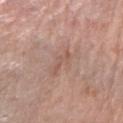lighting = white-light illumination
image = 15 mm crop, total-body photography
patient = female, aged 58–62
size = ~3 mm (longest diameter)
automated lesion analysis = a lesion area of about 2.5 mm², an eccentricity of roughly 0.95, and two-axis asymmetry of about 0.55; an average lesion color of about L≈55 a*≈20 b*≈26 (CIELAB), roughly 6 lightness units darker than nearby skin, and a normalized border contrast of about 4.5; a border-irregularity index near 7/10, a within-lesion color-variation index near 0/10, and peripheral color asymmetry of about 0; a classifier nevus-likeness of about 0/100
anatomic site = the right forearm